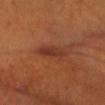biopsy_status: not biopsied; imaged during a skin examination
patient:
  sex: male
  age_approx: 60
lesion_size:
  long_diameter_mm_approx: 4.5
image:
  source: total-body photography crop
  field_of_view_mm: 15
lighting: cross-polarized
site: head or neck
automated_metrics:
  area_mm2_approx: 6.0
  eccentricity: 0.9
  shape_asymmetry: 0.25
  cielab_L: 34
  cielab_a: 25
  cielab_b: 28
  vs_skin_darker_L: 7.0
  vs_skin_contrast_norm: 7.5